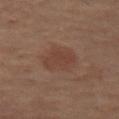Part of a total-body skin-imaging series; this lesion was reviewed on a skin check and was not flagged for biopsy. The lesion is on the leg. Measured at roughly 4.5 mm in maximum diameter. This is a cross-polarized tile. A female subject, roughly 65 years of age. The total-body-photography lesion software estimated a mean CIELAB color near L≈40 a*≈19 b*≈25, roughly 6 lightness units darker than nearby skin, and a lesion-to-skin contrast of about 5 (normalized; higher = more distinct). It also reported border irregularity of about 2 on a 0–10 scale, a within-lesion color-variation index near 3/10, and radial color variation of about 1. It also reported an automated nevus-likeness rating near 60 out of 100 and a lesion-detection confidence of about 100/100. A 15 mm close-up tile from a total-body photography series done for melanoma screening.